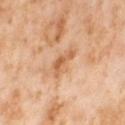Part of a total-body skin-imaging series; this lesion was reviewed on a skin check and was not flagged for biopsy.
A 15 mm crop from a total-body photograph taken for skin-cancer surveillance.
A female patient, aged around 55.
The lesion is located on the leg.